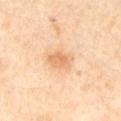- diameter: about 3.5 mm
- illumination: cross-polarized
- site: the right thigh
- subject: female, aged approximately 50
- image source: 15 mm crop, total-body photography
- automated lesion analysis: a border-irregularity rating of about 2.5/10, a within-lesion color-variation index near 2.5/10, and a peripheral color-asymmetry measure near 1; an automated nevus-likeness rating near 55 out of 100